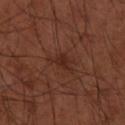Case summary:
– biopsy status: no biopsy performed (imaged during a skin exam)
– acquisition: ~15 mm crop, total-body skin-cancer survey
– patient: male, in their 50s
– body site: the left arm